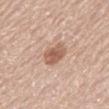| feature | finding |
|---|---|
| workup | no biopsy performed (imaged during a skin exam) |
| subject | male, in their 60s |
| size | ≈3 mm |
| body site | the mid back |
| illumination | white-light |
| image | ~15 mm tile from a whole-body skin photo |